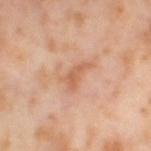Located on the left thigh. The subject is a female roughly 55 years of age. The lesion's longest dimension is about 3.5 mm. Automated tile analysis of the lesion measured an average lesion color of about L≈62 a*≈24 b*≈35 (CIELAB), about 8 CIELAB-L* units darker than the surrounding skin, and a normalized border contrast of about 6. The software also gave an automated nevus-likeness rating near 0 out of 100 and lesion-presence confidence of about 100/100. Cropped from a whole-body photographic skin survey; the tile spans about 15 mm.